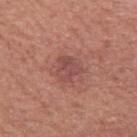notes: catalogued during a skin exam; not biopsied | illumination: white-light | image source: ~15 mm crop, total-body skin-cancer survey | lesion size: ~3 mm (longest diameter) | patient: male, aged approximately 65 | location: the left upper arm.